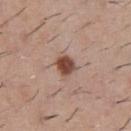workup = no biopsy performed (imaged during a skin exam)
body site = the chest
lesion size = about 2.5 mm
image = total-body-photography crop, ~15 mm field of view
subject = male, aged 28 to 32